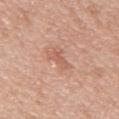The lesion was tiled from a total-body skin photograph and was not biopsied. Located on the back. Automated image analysis of the tile measured lesion-presence confidence of about 100/100. The patient is a male about 50 years old. A region of skin cropped from a whole-body photographic capture, roughly 15 mm wide.